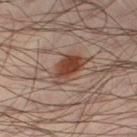Captured during whole-body skin photography for melanoma surveillance; the lesion was not biopsied. From the leg. A region of skin cropped from a whole-body photographic capture, roughly 15 mm wide. A male patient roughly 40 years of age. The tile uses cross-polarized illumination. Measured at roughly 4.5 mm in maximum diameter.